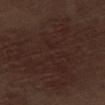This lesion was catalogued during total-body skin photography and was not selected for biopsy.